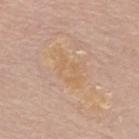follow-up — no biopsy performed (imaged during a skin exam) | tile lighting — white-light illumination | subject — female, aged 68–72 | lesion diameter — ≈4.5 mm | acquisition — ~15 mm crop, total-body skin-cancer survey | image-analysis metrics — an area of roughly 10 mm² and two-axis asymmetry of about 0.35; a lesion color around L≈64 a*≈17 b*≈34 in CIELAB, about 4 CIELAB-L* units darker than the surrounding skin, and a normalized lesion–skin contrast near 4.5 | anatomic site — the mid back.